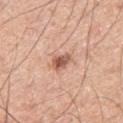No biopsy was performed on this lesion — it was imaged during a full skin examination and was not determined to be concerning. On the right thigh. Cropped from a total-body skin-imaging series; the visible field is about 15 mm. The recorded lesion diameter is about 2.5 mm. The lesion-visualizer software estimated border irregularity of about 3 on a 0–10 scale and a peripheral color-asymmetry measure near 1.5. And it measured an automated nevus-likeness rating near 80 out of 100 and a lesion-detection confidence of about 100/100. The patient is a male aged approximately 55.